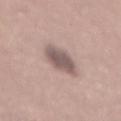biopsy_status: not biopsied; imaged during a skin examination
patient:
  sex: male
  age_approx: 40
lesion_size:
  long_diameter_mm_approx: 4.5
image:
  source: total-body photography crop
  field_of_view_mm: 15
lighting: white-light
site: mid back